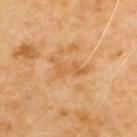Impression:
No biopsy was performed on this lesion — it was imaged during a full skin examination and was not determined to be concerning.
Context:
Cropped from a total-body skin-imaging series; the visible field is about 15 mm. The lesion is on the upper back. A male subject, aged approximately 70. The total-body-photography lesion software estimated an average lesion color of about L≈59 a*≈22 b*≈42 (CIELAB), a lesion–skin lightness drop of about 7, and a normalized lesion–skin contrast near 5. The recorded lesion diameter is about 5 mm. Imaged with cross-polarized lighting.